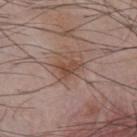Clinical impression: Recorded during total-body skin imaging; not selected for excision or biopsy. Image and clinical context: On the chest. A region of skin cropped from a whole-body photographic capture, roughly 15 mm wide. A male subject, aged approximately 55.